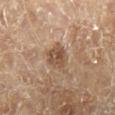biopsy_status: not biopsied; imaged during a skin examination